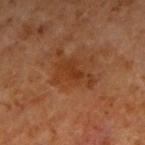This is a cross-polarized tile. A male subject, approximately 60 years of age. Cropped from a total-body skin-imaging series; the visible field is about 15 mm. The lesion-visualizer software estimated an area of roughly 9.5 mm², a shape eccentricity near 0.8, and two-axis asymmetry of about 0.45. And it measured an average lesion color of about L≈35 a*≈23 b*≈33 (CIELAB). The software also gave border irregularity of about 5.5 on a 0–10 scale, a color-variation rating of about 3/10, and a peripheral color-asymmetry measure near 1. The lesion is located on the right upper arm. The lesion's longest dimension is about 4.5 mm.